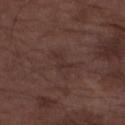The lesion was photographed on a routine skin check and not biopsied; there is no pathology result.
Imaged with white-light lighting.
A region of skin cropped from a whole-body photographic capture, roughly 15 mm wide.
The lesion's longest dimension is about 3 mm.
A male patient, approximately 75 years of age.
The lesion is located on the right forearm.